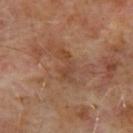No biopsy was performed on this lesion — it was imaged during a full skin examination and was not determined to be concerning. About 4 mm across. Imaged with cross-polarized lighting. A region of skin cropped from a whole-body photographic capture, roughly 15 mm wide. A male patient approximately 65 years of age. The lesion is on the upper back.